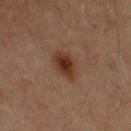Clinical impression: The lesion was tiled from a total-body skin photograph and was not biopsied. Clinical summary: Located on the right upper arm. The total-body-photography lesion software estimated a lesion–skin lightness drop of about 8. The software also gave border irregularity of about 2 on a 0–10 scale, a within-lesion color-variation index near 3/10, and radial color variation of about 1. The lesion's longest dimension is about 4 mm. A 15 mm crop from a total-body photograph taken for skin-cancer surveillance. This is a cross-polarized tile. The patient is a male aged around 60.